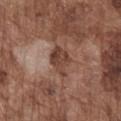The lesion was tiled from a total-body skin photograph and was not biopsied. A male subject, in their mid- to late 70s. The tile uses white-light illumination. From the chest. About 3.5 mm across. This image is a 15 mm lesion crop taken from a total-body photograph.